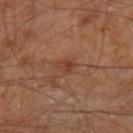biopsy status = total-body-photography surveillance lesion; no biopsy
lighting = cross-polarized illumination
site = the right leg
acquisition = ~15 mm tile from a whole-body skin photo
lesion diameter = ~2.5 mm (longest diameter)
patient = male, aged approximately 60